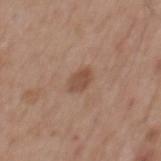Q: Was this lesion biopsied?
A: total-body-photography surveillance lesion; no biopsy
Q: Lesion location?
A: the mid back
Q: How was this image acquired?
A: ~15 mm tile from a whole-body skin photo
Q: Patient demographics?
A: male, about 55 years old
Q: How large is the lesion?
A: about 3 mm